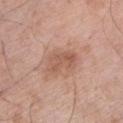Part of a total-body skin-imaging series; this lesion was reviewed on a skin check and was not flagged for biopsy.
A male subject, roughly 80 years of age.
A 15 mm crop from a total-body photograph taken for skin-cancer surveillance.
The recorded lesion diameter is about 4 mm.
The lesion-visualizer software estimated a footprint of about 7.5 mm², a shape eccentricity near 0.8, and a shape-asymmetry score of about 0.3 (0 = symmetric).
The lesion is on the left lower leg.